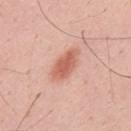| feature | finding |
|---|---|
| notes | imaged on a skin check; not biopsied |
| site | the upper back |
| TBP lesion metrics | a lesion area of about 7 mm², an eccentricity of roughly 0.85, and a shape-asymmetry score of about 0.2 (0 = symmetric); a lesion-to-skin contrast of about 8 (normalized; higher = more distinct); a border-irregularity index near 2/10 and internal color variation of about 2.5 on a 0–10 scale; a detector confidence of about 100 out of 100 that the crop contains a lesion |
| image source | ~15 mm tile from a whole-body skin photo |
| patient | male, in their mid- to late 30s |
| illumination | white-light illumination |